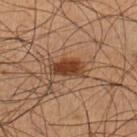The lesion was tiled from a total-body skin photograph and was not biopsied. The tile uses cross-polarized illumination. A lesion tile, about 15 mm wide, cut from a 3D total-body photograph. A male patient, aged 38 to 42. On the left lower leg.